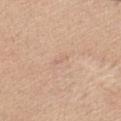| field | value |
|---|---|
| workup | imaged on a skin check; not biopsied |
| subject | female, approximately 45 years of age |
| illumination | white-light |
| imaging modality | ~15 mm tile from a whole-body skin photo |
| image-analysis metrics | a classifier nevus-likeness of about 0/100 and a detector confidence of about 100 out of 100 that the crop contains a lesion |
| location | the left upper arm |